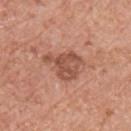This lesion was catalogued during total-body skin photography and was not selected for biopsy. A male patient aged 68 to 72. On the chest. A 15 mm crop from a total-body photograph taken for skin-cancer surveillance.